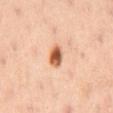  biopsy_status: not biopsied; imaged during a skin examination
  patient:
    sex: male
    age_approx: 55
  image:
    source: total-body photography crop
    field_of_view_mm: 15
  lighting: cross-polarized
  lesion_size:
    long_diameter_mm_approx: 3.0
  automated_metrics:
    area_mm2_approx: 4.5
    eccentricity: 0.7
    shape_asymmetry: 0.25
    cielab_L: 58
    cielab_a: 25
    cielab_b: 37
    vs_skin_darker_L: 18.0
    vs_skin_contrast_norm: 11.0
    border_irregularity_0_10: 2.0
    color_variation_0_10: 6.0
    peripheral_color_asymmetry: 1.5
    lesion_detection_confidence_0_100: 100
  site: back